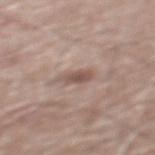Findings:
• notes — no biopsy performed (imaged during a skin exam)
• imaging modality — 15 mm crop, total-body photography
• lesion diameter — ~3.5 mm (longest diameter)
• patient — male, roughly 60 years of age
• body site — the mid back
• automated lesion analysis — a lesion area of about 4 mm², an outline eccentricity of about 0.9 (0 = round, 1 = elongated), and a symmetry-axis asymmetry near 0.25; an automated nevus-likeness rating near 0 out of 100 and lesion-presence confidence of about 95/100
• tile lighting — white-light illumination